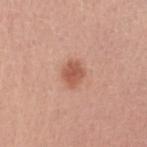The lesion is on the right upper arm.
The patient is a female aged approximately 50.
Cropped from a whole-body photographic skin survey; the tile spans about 15 mm.
Approximately 3 mm at its widest.
This is a white-light tile.
Automated tile analysis of the lesion measured a shape-asymmetry score of about 0.25 (0 = symmetric). It also reported border irregularity of about 2 on a 0–10 scale.